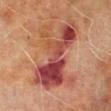No biopsy was performed on this lesion — it was imaged during a full skin examination and was not determined to be concerning.
The lesion's longest dimension is about 9 mm.
Located on the left lower leg.
Cropped from a whole-body photographic skin survey; the tile spans about 15 mm.
A male subject, in their 70s.